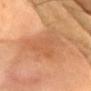Impression:
Recorded during total-body skin imaging; not selected for excision or biopsy.